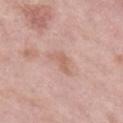Part of a total-body skin-imaging series; this lesion was reviewed on a skin check and was not flagged for biopsy. An algorithmic analysis of the crop reported a footprint of about 4.5 mm² and two-axis asymmetry of about 0.5. The analysis additionally found a mean CIELAB color near L≈62 a*≈20 b*≈27 and a lesion-to-skin contrast of about 5.5 (normalized; higher = more distinct). A lesion tile, about 15 mm wide, cut from a 3D total-body photograph. Imaged with white-light lighting. About 3.5 mm across. A female patient about 70 years old. From the leg.